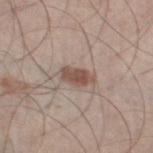Assessment: Captured during whole-body skin photography for melanoma surveillance; the lesion was not biopsied. Clinical summary: A 15 mm crop from a total-body photograph taken for skin-cancer surveillance. On the left thigh. A male subject aged 58 to 62.